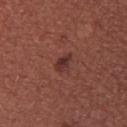{"biopsy_status": "not biopsied; imaged during a skin examination", "lesion_size": {"long_diameter_mm_approx": 2.5}, "image": {"source": "total-body photography crop", "field_of_view_mm": 15}, "lighting": "white-light", "site": "back", "patient": {"sex": "female", "age_approx": 25}}